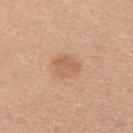This image is a 15 mm lesion crop taken from a total-body photograph. The tile uses white-light illumination. Located on the upper back. The patient is a female aged 38–42. An algorithmic analysis of the crop reported a lesion area of about 5.5 mm², an eccentricity of roughly 0.7, and a shape-asymmetry score of about 0.4 (0 = symmetric). And it measured a classifier nevus-likeness of about 35/100 and a lesion-detection confidence of about 100/100.